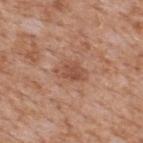Imaged during a routine full-body skin examination; the lesion was not biopsied and no histopathology is available. A male patient aged approximately 65. Located on the upper back. Captured under white-light illumination. Measured at roughly 3.5 mm in maximum diameter. Cropped from a whole-body photographic skin survey; the tile spans about 15 mm.